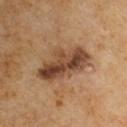{
  "biopsy_status": "not biopsied; imaged during a skin examination",
  "image": {
    "source": "total-body photography crop",
    "field_of_view_mm": 15
  },
  "patient": {
    "sex": "male",
    "age_approx": 60
  },
  "site": "upper back"
}